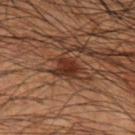  biopsy_status: not biopsied; imaged during a skin examination
  patient:
    sex: male
    age_approx: 50
  lighting: cross-polarized
  automated_metrics:
    area_mm2_approx: 7.0
    shape_asymmetry: 0.3
    cielab_L: 24
    cielab_a: 17
    cielab_b: 22
    vs_skin_darker_L: 8.0
    vs_skin_contrast_norm: 9.0
  site: left forearm
  image:
    source: total-body photography crop
    field_of_view_mm: 15